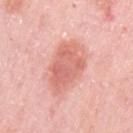Part of a total-body skin-imaging series; this lesion was reviewed on a skin check and was not flagged for biopsy.
Located on the arm.
This image is a 15 mm lesion crop taken from a total-body photograph.
A female patient aged 63 to 67.
Measured at roughly 6.5 mm in maximum diameter.